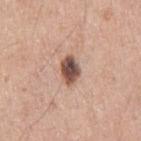Notes:
• notes: total-body-photography surveillance lesion; no biopsy
• patient: male, aged around 45
• image source: ~15 mm tile from a whole-body skin photo
• anatomic site: the right upper arm
• lighting: white-light illumination